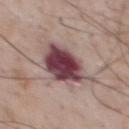biopsy_status: not biopsied; imaged during a skin examination
lighting: white-light
automated_metrics:
  area_mm2_approx: 23.0
  shape_asymmetry: 0.25
  cielab_L: 46
  cielab_a: 21
  cielab_b: 16
  nevus_likeness_0_100: 50
  lesion_detection_confidence_0_100: 100
site: upper back
lesion_size:
  long_diameter_mm_approx: 7.0
patient:
  sex: male
  age_approx: 55
image:
  source: total-body photography crop
  field_of_view_mm: 15